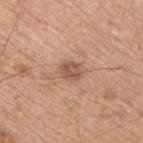Clinical impression:
Part of a total-body skin-imaging series; this lesion was reviewed on a skin check and was not flagged for biopsy.
Context:
The lesion is on the right upper arm. Captured under white-light illumination. Longest diameter approximately 2.5 mm. This image is a 15 mm lesion crop taken from a total-body photograph. A male subject aged 53 to 57.